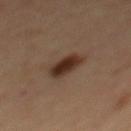Q: What did automated image analysis measure?
A: a shape eccentricity near 0.75 and a symmetry-axis asymmetry near 0.15; a lesion color around L≈30 a*≈17 b*≈24 in CIELAB, roughly 13 lightness units darker than nearby skin, and a lesion-to-skin contrast of about 12 (normalized; higher = more distinct); border irregularity of about 1.5 on a 0–10 scale, a within-lesion color-variation index near 3.5/10, and peripheral color asymmetry of about 1
Q: Lesion location?
A: the mid back
Q: Illumination type?
A: cross-polarized illumination
Q: Patient demographics?
A: male, aged around 60
Q: How was this image acquired?
A: total-body-photography crop, ~15 mm field of view